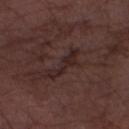biopsy status — no biopsy performed (imaged during a skin exam) | automated metrics — an area of roughly 6 mm², a shape eccentricity near 0.95, and a symmetry-axis asymmetry near 0.45; a lesion color around L≈24 a*≈16 b*≈16 in CIELAB, a lesion–skin lightness drop of about 6, and a lesion-to-skin contrast of about 7 (normalized; higher = more distinct); a border-irregularity rating of about 6.5/10, a color-variation rating of about 1/10, and peripheral color asymmetry of about 0 | site — the right forearm | imaging modality — ~15 mm tile from a whole-body skin photo | patient — male, aged 73–77 | tile lighting — white-light | diameter — about 5 mm.